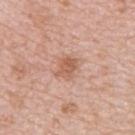biopsy status: catalogued during a skin exam; not biopsied | image-analysis metrics: an average lesion color of about L≈59 a*≈22 b*≈31 (CIELAB), about 9 CIELAB-L* units darker than the surrounding skin, and a lesion-to-skin contrast of about 6.5 (normalized; higher = more distinct); border irregularity of about 2.5 on a 0–10 scale, internal color variation of about 3 on a 0–10 scale, and a peripheral color-asymmetry measure near 1; a nevus-likeness score of about 35/100 and lesion-presence confidence of about 100/100 | diameter: about 3 mm | patient: female, approximately 45 years of age | anatomic site: the back | lighting: white-light | image source: ~15 mm tile from a whole-body skin photo.